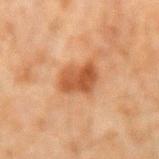Background:
The lesion is located on the right forearm. The tile uses cross-polarized illumination. A close-up tile cropped from a whole-body skin photograph, about 15 mm across. The patient is a male roughly 45 years of age.